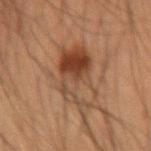A male subject roughly 35 years of age.
Measured at roughly 8 mm in maximum diameter.
This image is a 15 mm lesion crop taken from a total-body photograph.
The lesion is located on the right forearm.